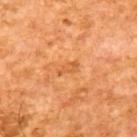Part of a total-body skin-imaging series; this lesion was reviewed on a skin check and was not flagged for biopsy.